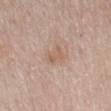Assessment: This lesion was catalogued during total-body skin photography and was not selected for biopsy. Clinical summary: A 15 mm crop from a total-body photograph taken for skin-cancer surveillance. The tile uses white-light illumination. A female patient, aged 63–67. Approximately 2.5 mm at its widest. The lesion is located on the back.